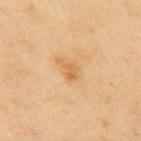{
  "biopsy_status": "not biopsied; imaged during a skin examination",
  "patient": {
    "sex": "male",
    "age_approx": 45
  },
  "site": "back",
  "lighting": "cross-polarized",
  "image": {
    "source": "total-body photography crop",
    "field_of_view_mm": 15
  },
  "lesion_size": {
    "long_diameter_mm_approx": 2.5
  }
}